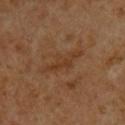Clinical impression:
This lesion was catalogued during total-body skin photography and was not selected for biopsy.
Acquisition and patient details:
The lesion is on the upper back. A region of skin cropped from a whole-body photographic capture, roughly 15 mm wide. The subject is a male approximately 60 years of age.